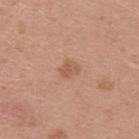Findings:
– workup: total-body-photography surveillance lesion; no biopsy
– imaging modality: total-body-photography crop, ~15 mm field of view
– location: the upper back
– subject: male, approximately 25 years of age
– size: ~2.5 mm (longest diameter)